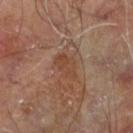biopsy status: no biopsy performed (imaged during a skin exam) | lighting: cross-polarized illumination | image: 15 mm crop, total-body photography | body site: the left lower leg | automated metrics: a lesion area of about 6 mm² and a shape-asymmetry score of about 0.25 (0 = symmetric); internal color variation of about 2.5 on a 0–10 scale and radial color variation of about 1 | patient: male, aged around 70 | diameter: ≈4 mm.